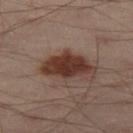Q: Is there a histopathology result?
A: imaged on a skin check; not biopsied
Q: What is the imaging modality?
A: ~15 mm tile from a whole-body skin photo
Q: How was the tile lit?
A: cross-polarized
Q: Patient demographics?
A: male, about 50 years old
Q: Lesion location?
A: the leg
Q: Lesion size?
A: ~5 mm (longest diameter)